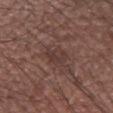Assessment:
No biopsy was performed on this lesion — it was imaged during a full skin examination and was not determined to be concerning.
Clinical summary:
The patient is a male roughly 55 years of age. Imaged with white-light lighting. An algorithmic analysis of the crop reported a lesion area of about 5.5 mm², a shape eccentricity near 0.6, and a symmetry-axis asymmetry near 0.25. And it measured a border-irregularity index near 2.5/10 and internal color variation of about 2 on a 0–10 scale. It also reported a nevus-likeness score of about 0/100 and a detector confidence of about 80 out of 100 that the crop contains a lesion. About 3 mm across. A lesion tile, about 15 mm wide, cut from a 3D total-body photograph. From the right forearm.